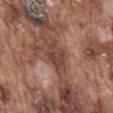No biopsy was performed on this lesion — it was imaged during a full skin examination and was not determined to be concerning. A 15 mm close-up tile from a total-body photography series done for melanoma screening. Longest diameter approximately 3 mm. This is a white-light tile. From the mid back. A male subject roughly 75 years of age.